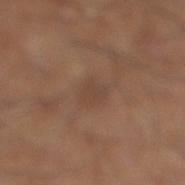- image · 15 mm crop, total-body photography
- site · the right lower leg
- lesion size · about 3 mm
- patient · male, aged approximately 60
- TBP lesion metrics · a lesion color around L≈43 a*≈17 b*≈27 in CIELAB, about 6 CIELAB-L* units darker than the surrounding skin, and a lesion-to-skin contrast of about 5 (normalized; higher = more distinct); a color-variation rating of about 2.5/10 and radial color variation of about 1; a classifier nevus-likeness of about 0/100 and lesion-presence confidence of about 100/100